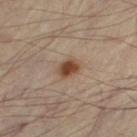notes — catalogued during a skin exam; not biopsied
location — the right thigh
image source — ~15 mm crop, total-body skin-cancer survey
patient — male, approximately 55 years of age